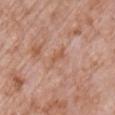Impression: Imaged during a routine full-body skin examination; the lesion was not biopsied and no histopathology is available.